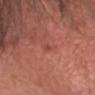notes = imaged on a skin check; not biopsied | illumination = white-light | anatomic site = the head or neck | subject = male, in their mid-40s | acquisition = ~15 mm crop, total-body skin-cancer survey | lesion diameter = ~1.5 mm (longest diameter).